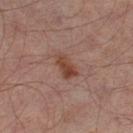| field | value |
|---|---|
| workup | no biopsy performed (imaged during a skin exam) |
| acquisition | 15 mm crop, total-body photography |
| subject | male, in their mid-60s |
| location | the left thigh |